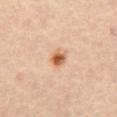Located on the front of the torso. A roughly 15 mm field-of-view crop from a total-body skin photograph. Measured at roughly 2.5 mm in maximum diameter. A female subject, roughly 65 years of age. Imaged with cross-polarized lighting.